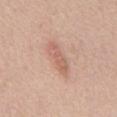Findings:
• notes · catalogued during a skin exam; not biopsied
• site · the abdomen
• subject · male, approximately 55 years of age
• image · ~15 mm tile from a whole-body skin photo